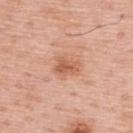Clinical summary:
On the upper back. Measured at roughly 2.5 mm in maximum diameter. A male patient, roughly 60 years of age. This image is a 15 mm lesion crop taken from a total-body photograph. Automated tile analysis of the lesion measured a classifier nevus-likeness of about 20/100 and a lesion-detection confidence of about 100/100. Imaged with white-light lighting.